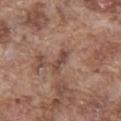Notes:
– notes · catalogued during a skin exam; not biopsied
– patient · male, roughly 75 years of age
– acquisition · ~15 mm tile from a whole-body skin photo
– body site · the abdomen